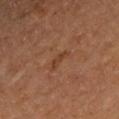Q: Is there a histopathology result?
A: total-body-photography surveillance lesion; no biopsy
Q: How large is the lesion?
A: ≈3 mm
Q: Patient demographics?
A: male, in their mid-60s
Q: What is the imaging modality?
A: ~15 mm crop, total-body skin-cancer survey
Q: What lighting was used for the tile?
A: cross-polarized illumination
Q: Lesion location?
A: the left upper arm
Q: Automated lesion metrics?
A: an area of roughly 3.5 mm², an outline eccentricity of about 0.9 (0 = round, 1 = elongated), and a symmetry-axis asymmetry near 0.4; a mean CIELAB color near L≈41 a*≈22 b*≈31, a lesion–skin lightness drop of about 6, and a normalized lesion–skin contrast near 5.5; a classifier nevus-likeness of about 0/100 and a detector confidence of about 100 out of 100 that the crop contains a lesion